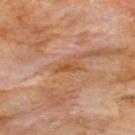Context:
Automated tile analysis of the lesion measured a lesion area of about 2.5 mm², an eccentricity of roughly 0.95, and a symmetry-axis asymmetry near 0.25. The software also gave a lesion color around L≈49 a*≈24 b*≈38 in CIELAB, about 8 CIELAB-L* units darker than the surrounding skin, and a lesion-to-skin contrast of about 8 (normalized; higher = more distinct). Captured under cross-polarized illumination. About 3 mm across. From the front of the torso. A lesion tile, about 15 mm wide, cut from a 3D total-body photograph. A male patient aged 58–62.